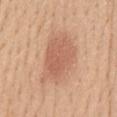Case summary:
- notes: no biopsy performed (imaged during a skin exam)
- lighting: white-light illumination
- anatomic site: the front of the torso
- image-analysis metrics: a footprint of about 21 mm², a shape eccentricity near 0.8, and a symmetry-axis asymmetry near 0.2
- image source: ~15 mm crop, total-body skin-cancer survey
- patient: female, about 45 years old